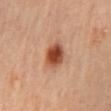Notes:
– notes — total-body-photography surveillance lesion; no biopsy
– anatomic site — the mid back
– automated lesion analysis — a lesion color around L≈49 a*≈28 b*≈35 in CIELAB, about 17 CIELAB-L* units darker than the surrounding skin, and a normalized lesion–skin contrast near 11.5; border irregularity of about 1.5 on a 0–10 scale and internal color variation of about 5.5 on a 0–10 scale; an automated nevus-likeness rating near 100 out of 100 and lesion-presence confidence of about 100/100
– image — total-body-photography crop, ~15 mm field of view
– lighting — cross-polarized
– subject — male, in their mid- to late 50s
– size — about 3 mm